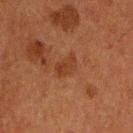Q: Was a biopsy performed?
A: catalogued during a skin exam; not biopsied
Q: Who is the patient?
A: male, aged 58 to 62
Q: What lighting was used for the tile?
A: cross-polarized
Q: Automated lesion metrics?
A: an area of roughly 5 mm², a shape eccentricity near 0.85, and a symmetry-axis asymmetry near 0.25; a peripheral color-asymmetry measure near 1
Q: What kind of image is this?
A: ~15 mm tile from a whole-body skin photo
Q: How large is the lesion?
A: ≈3.5 mm
Q: What is the anatomic site?
A: the head or neck